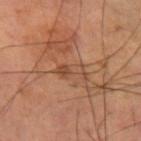lesion diameter=≈3.5 mm | location=the left forearm | tile lighting=cross-polarized | imaging modality=total-body-photography crop, ~15 mm field of view | patient=male, aged approximately 60.